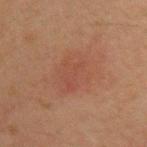No biopsy was performed on this lesion — it was imaged during a full skin examination and was not determined to be concerning. The lesion is located on the left upper arm. A male subject, aged 43–47. A 15 mm crop from a total-body photograph taken for skin-cancer surveillance. The lesion's longest dimension is about 6.5 mm. Captured under cross-polarized illumination.